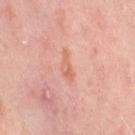Notes:
- notes: catalogued during a skin exam; not biopsied
- lesion diameter: ≈4 mm
- automated metrics: border irregularity of about 5 on a 0–10 scale and a color-variation rating of about 2/10
- acquisition: ~15 mm crop, total-body skin-cancer survey
- patient: female, approximately 50 years of age
- anatomic site: the left thigh
- illumination: cross-polarized illumination